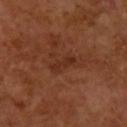Acquisition and patient details: A female subject approximately 50 years of age. A region of skin cropped from a whole-body photographic capture, roughly 15 mm wide. The lesion is located on the right forearm. This is a cross-polarized tile. The total-body-photography lesion software estimated a lesion area of about 3.5 mm². The analysis additionally found border irregularity of about 4.5 on a 0–10 scale, a within-lesion color-variation index near 0/10, and radial color variation of about 0. About 3.5 mm across.